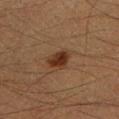Notes:
– notes: catalogued during a skin exam; not biopsied
– patient: male, roughly 40 years of age
– image: total-body-photography crop, ~15 mm field of view
– size: ≈3 mm
– body site: the left lower leg